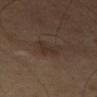Background:
Automated image analysis of the tile measured an area of roughly 5.5 mm², a shape eccentricity near 0.55, and a symmetry-axis asymmetry near 0.45. It also reported an average lesion color of about L≈29 a*≈12 b*≈20 (CIELAB) and a normalized border contrast of about 6. It also reported border irregularity of about 5 on a 0–10 scale. The software also gave a nevus-likeness score of about 5/100 and lesion-presence confidence of about 100/100. The recorded lesion diameter is about 3 mm. This is a cross-polarized tile. A male subject, roughly 65 years of age. Cropped from a whole-body photographic skin survey; the tile spans about 15 mm.